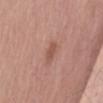This lesion was catalogued during total-body skin photography and was not selected for biopsy. Captured under white-light illumination. Located on the abdomen. A male subject, roughly 60 years of age. A lesion tile, about 15 mm wide, cut from a 3D total-body photograph.